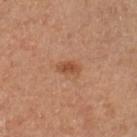Case summary:
- follow-up — no biopsy performed (imaged during a skin exam)
- subject — male, aged around 65
- image — total-body-photography crop, ~15 mm field of view
- body site — the left lower leg